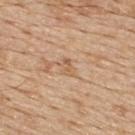Q: Was a biopsy performed?
A: imaged on a skin check; not biopsied
Q: Automated lesion metrics?
A: a symmetry-axis asymmetry near 0.35; a lesion color around L≈60 a*≈18 b*≈35 in CIELAB, about 7 CIELAB-L* units darker than the surrounding skin, and a normalized border contrast of about 5.5; a classifier nevus-likeness of about 0/100 and lesion-presence confidence of about 80/100
Q: What lighting was used for the tile?
A: white-light
Q: What is the imaging modality?
A: ~15 mm crop, total-body skin-cancer survey
Q: What are the patient's age and sex?
A: male, aged 68–72
Q: Lesion location?
A: the upper back
Q: How large is the lesion?
A: about 2.5 mm